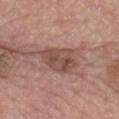Q: Was a biopsy performed?
A: total-body-photography surveillance lesion; no biopsy
Q: Illumination type?
A: white-light illumination
Q: How large is the lesion?
A: ≈5 mm
Q: What is the imaging modality?
A: ~15 mm crop, total-body skin-cancer survey
Q: Where on the body is the lesion?
A: the chest
Q: Who is the patient?
A: female, aged 63–67